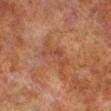Findings:
– workup — imaged on a skin check; not biopsied
– lesion diameter — about 5 mm
– image — ~15 mm crop, total-body skin-cancer survey
– subject — male, aged around 70
– illumination — cross-polarized
– body site — the right lower leg